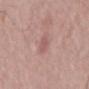No biopsy was performed on this lesion — it was imaged during a full skin examination and was not determined to be concerning. The lesion is on the mid back. Captured under white-light illumination. About 2.5 mm across. The patient is a male approximately 65 years of age. A 15 mm close-up extracted from a 3D total-body photography capture.